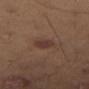biopsy status = no biopsy performed (imaged during a skin exam)
illumination = cross-polarized
imaging modality = 15 mm crop, total-body photography
patient = male, approximately 45 years of age
diameter = ≈2.5 mm
body site = the left thigh